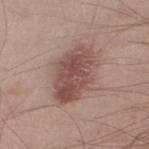Assessment:
This lesion was catalogued during total-body skin photography and was not selected for biopsy.
Clinical summary:
A male patient in their mid- to late 30s. The lesion is located on the leg. Imaged with white-light lighting. A 15 mm crop from a total-body photograph taken for skin-cancer surveillance. Measured at roughly 6.5 mm in maximum diameter. Automated tile analysis of the lesion measured a lesion area of about 21 mm², a shape eccentricity near 0.8, and a symmetry-axis asymmetry near 0.15. The analysis additionally found a border-irregularity index near 2/10 and radial color variation of about 2. And it measured an automated nevus-likeness rating near 80 out of 100 and lesion-presence confidence of about 100/100.